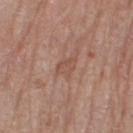notes: no biopsy performed (imaged during a skin exam)
subject: female, about 75 years old
TBP lesion metrics: a footprint of about 4 mm², an eccentricity of roughly 0.6, and a symmetry-axis asymmetry near 0.25; a border-irregularity index near 2.5/10, internal color variation of about 1.5 on a 0–10 scale, and peripheral color asymmetry of about 0.5
diameter: ~2.5 mm (longest diameter)
imaging modality: 15 mm crop, total-body photography
site: the leg